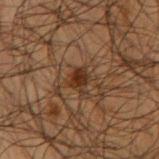  biopsy_status: not biopsied; imaged during a skin examination
  site: right upper arm
  image:
    source: total-body photography crop
    field_of_view_mm: 15
  patient:
    sex: male
    age_approx: 50